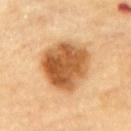Captured during whole-body skin photography for melanoma surveillance; the lesion was not biopsied. A lesion tile, about 15 mm wide, cut from a 3D total-body photograph. The recorded lesion diameter is about 6 mm. Captured under cross-polarized illumination. The lesion is located on the upper back. A male patient in their mid- to late 80s or older.